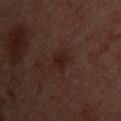Notes:
- follow-up · no biopsy performed (imaged during a skin exam)
- tile lighting · cross-polarized illumination
- lesion size · about 3 mm
- location · the chest
- TBP lesion metrics · a within-lesion color-variation index near 2/10 and a peripheral color-asymmetry measure near 0.5; a classifier nevus-likeness of about 25/100 and lesion-presence confidence of about 100/100
- subject · female, roughly 55 years of age
- imaging modality · ~15 mm crop, total-body skin-cancer survey